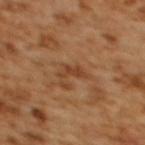Acquisition and patient details: The patient is a female aged approximately 55. A 15 mm crop from a total-body photograph taken for skin-cancer surveillance. The lesion is located on the upper back.